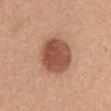No biopsy was performed on this lesion — it was imaged during a full skin examination and was not determined to be concerning. Cropped from a whole-body photographic skin survey; the tile spans about 15 mm. On the chest. About 5 mm across. The subject is a female aged 53–57.